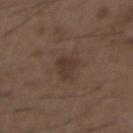– notes: total-body-photography surveillance lesion; no biopsy
– patient: male, about 50 years old
– image source: total-body-photography crop, ~15 mm field of view
– lesion size: about 3 mm
– location: the chest
– tile lighting: white-light